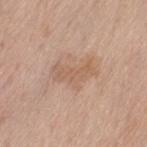Part of a total-body skin-imaging series; this lesion was reviewed on a skin check and was not flagged for biopsy. Imaged with white-light lighting. On the lower back. A female patient, approximately 65 years of age. Cropped from a total-body skin-imaging series; the visible field is about 15 mm.